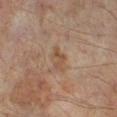biopsy status: imaged on a skin check; not biopsied
lesion size: ~3 mm (longest diameter)
image-analysis metrics: a lesion area of about 3 mm², an eccentricity of roughly 0.85, and a shape-asymmetry score of about 0.5 (0 = symmetric); a border-irregularity rating of about 5.5/10 and a color-variation rating of about 0/10
anatomic site: the right lower leg
patient: male, approximately 65 years of age
imaging modality: ~15 mm crop, total-body skin-cancer survey
illumination: cross-polarized